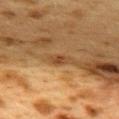This lesion was catalogued during total-body skin photography and was not selected for biopsy. On the upper back. The lesion's longest dimension is about 2.5 mm. The tile uses cross-polarized illumination. A region of skin cropped from a whole-body photographic capture, roughly 15 mm wide. The patient is a female approximately 40 years of age.